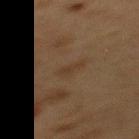Q: Was this lesion biopsied?
A: total-body-photography surveillance lesion; no biopsy
Q: What did automated image analysis measure?
A: a lesion area of about 3 mm² and a shape eccentricity near 0.85; a mean CIELAB color near L≈31 a*≈13 b*≈25, roughly 4 lightness units darker than nearby skin, and a normalized border contrast of about 5; a classifier nevus-likeness of about 5/100 and lesion-presence confidence of about 100/100
Q: What is the imaging modality?
A: ~15 mm crop, total-body skin-cancer survey
Q: How large is the lesion?
A: about 2.5 mm
Q: What lighting was used for the tile?
A: cross-polarized illumination
Q: Who is the patient?
A: female, roughly 40 years of age
Q: Where on the body is the lesion?
A: the mid back